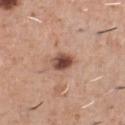Q: Was a biopsy performed?
A: total-body-photography surveillance lesion; no biopsy
Q: What is the lesion's diameter?
A: ≈3 mm
Q: What are the patient's age and sex?
A: male, in their mid- to late 40s
Q: Where on the body is the lesion?
A: the chest
Q: How was the tile lit?
A: white-light
Q: What is the imaging modality?
A: total-body-photography crop, ~15 mm field of view